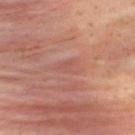  biopsy_status: not biopsied; imaged during a skin examination
  site: upper back
  automated_metrics:
    area_mm2_approx: 5.0
    shape_asymmetry: 0.45
    cielab_L: 52
    cielab_a: 24
    cielab_b: 25
    vs_skin_darker_L: 4.0
    vs_skin_contrast_norm: 3.5
    border_irregularity_0_10: 5.5
    color_variation_0_10: 1.5
    peripheral_color_asymmetry: 0.5
  patient:
    sex: male
    age_approx: 35
  image:
    source: total-body photography crop
    field_of_view_mm: 15
  lighting: cross-polarized